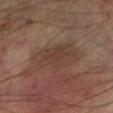The lesion was tiled from a total-body skin photograph and was not biopsied.
A male patient aged 68–72.
This is a cross-polarized tile.
About 6 mm across.
A close-up tile cropped from a whole-body skin photograph, about 15 mm across.
On the left lower leg.